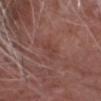Clinical impression: This lesion was catalogued during total-body skin photography and was not selected for biopsy. Clinical summary: A 15 mm close-up tile from a total-body photography series done for melanoma screening. This is a white-light tile. The lesion's longest dimension is about 2.5 mm. The subject is a male about 70 years old. On the head or neck.